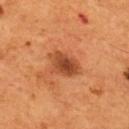Impression:
The lesion was photographed on a routine skin check and not biopsied; there is no pathology result.
Image and clinical context:
This image is a 15 mm lesion crop taken from a total-body photograph. Located on the back. A male subject approximately 55 years of age. The tile uses cross-polarized illumination.